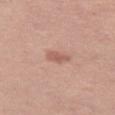The lesion was photographed on a routine skin check and not biopsied; there is no pathology result. A female patient roughly 50 years of age. A lesion tile, about 15 mm wide, cut from a 3D total-body photograph. Located on the left thigh. An algorithmic analysis of the crop reported a footprint of about 3 mm² and a shape eccentricity near 0.85. And it measured a lesion color around L≈58 a*≈24 b*≈26 in CIELAB, about 9 CIELAB-L* units darker than the surrounding skin, and a normalized lesion–skin contrast near 6.5. And it measured a classifier nevus-likeness of about 55/100 and a lesion-detection confidence of about 100/100.